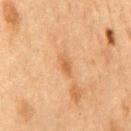Clinical impression:
Captured during whole-body skin photography for melanoma surveillance; the lesion was not biopsied.
Acquisition and patient details:
A male subject in their mid- to late 70s. On the front of the torso. The lesion's longest dimension is about 3 mm. Imaged with cross-polarized lighting. Automated tile analysis of the lesion measured a footprint of about 3 mm² and a shape-asymmetry score of about 0.3 (0 = symmetric). And it measured a lesion color around L≈49 a*≈19 b*≈34 in CIELAB, a lesion–skin lightness drop of about 7, and a normalized border contrast of about 6. And it measured a border-irregularity rating of about 3.5/10 and a within-lesion color-variation index near 1/10. A 15 mm close-up tile from a total-body photography series done for melanoma screening.